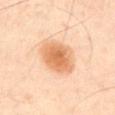The lesion was photographed on a routine skin check and not biopsied; there is no pathology result.